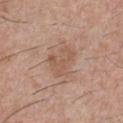The lesion was photographed on a routine skin check and not biopsied; there is no pathology result. Cropped from a total-body skin-imaging series; the visible field is about 15 mm. Located on the chest. A male subject, aged approximately 30.